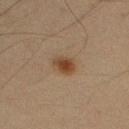notes: imaged on a skin check; not biopsied | site: the right upper arm | subject: male, aged around 35 | image source: total-body-photography crop, ~15 mm field of view.